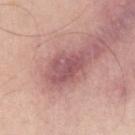Clinical impression:
Recorded during total-body skin imaging; not selected for excision or biopsy.
Clinical summary:
About 5 mm across. A 15 mm close-up tile from a total-body photography series done for melanoma screening. A male patient, roughly 50 years of age. Located on the right upper arm.